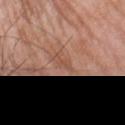Impression: Captured during whole-body skin photography for melanoma surveillance; the lesion was not biopsied. Image and clinical context: The subject is a male roughly 60 years of age. A 15 mm close-up extracted from a 3D total-body photography capture. Located on the left forearm. The recorded lesion diameter is about 3 mm.